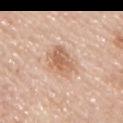Approximately 4.5 mm at its widest.
A male subject aged 43–47.
On the chest.
Captured under white-light illumination.
A 15 mm close-up tile from a total-body photography series done for melanoma screening.